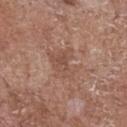notes — imaged on a skin check; not biopsied | imaging modality — total-body-photography crop, ~15 mm field of view | patient — male, in their 70s | diameter — ≈4.5 mm | lighting — white-light | site — the chest | automated lesion analysis — a within-lesion color-variation index near 3/10 and radial color variation of about 1; a nevus-likeness score of about 0/100 and lesion-presence confidence of about 100/100.